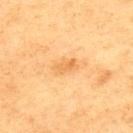<record>
  <image>
    <source>total-body photography crop</source>
    <field_of_view_mm>15</field_of_view_mm>
  </image>
  <site>upper back</site>
  <patient>
    <sex>male</sex>
    <age_approx>75</age_approx>
  </patient>
  <lighting>cross-polarized</lighting>
  <lesion_size>
    <long_diameter_mm_approx>3.0</long_diameter_mm_approx>
  </lesion_size>
</record>